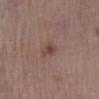Part of a total-body skin-imaging series; this lesion was reviewed on a skin check and was not flagged for biopsy. The subject is a female aged around 65. Cropped from a whole-body photographic skin survey; the tile spans about 15 mm. About 2.5 mm across. From the left lower leg. The tile uses white-light illumination.